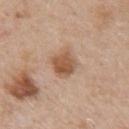{
  "biopsy_status": "not biopsied; imaged during a skin examination"
}